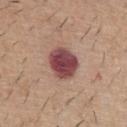* workup — imaged on a skin check; not biopsied
* imaging modality — ~15 mm tile from a whole-body skin photo
* subject — male, aged around 60
* lighting — white-light illumination
* site — the abdomen
* diameter — ≈4 mm